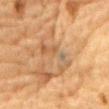No biopsy was performed on this lesion — it was imaged during a full skin examination and was not determined to be concerning.